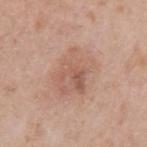{"biopsy_status": "not biopsied; imaged during a skin examination", "site": "left upper arm", "lesion_size": {"long_diameter_mm_approx": 5.0}, "image": {"source": "total-body photography crop", "field_of_view_mm": 15}, "patient": {"sex": "male", "age_approx": 40}, "automated_metrics": {"cielab_L": 59, "cielab_a": 20, "cielab_b": 28, "vs_skin_darker_L": 8.0, "vs_skin_contrast_norm": 5.0, "border_irregularity_0_10": 3.0, "color_variation_0_10": 5.5}, "lighting": "white-light"}